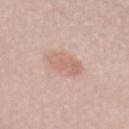workup — catalogued during a skin exam; not biopsied
TBP lesion metrics — a lesion area of about 8 mm², an eccentricity of roughly 0.65, and a shape-asymmetry score of about 0.25 (0 = symmetric); a color-variation rating of about 3/10 and a peripheral color-asymmetry measure near 1; a nevus-likeness score of about 10/100 and a lesion-detection confidence of about 100/100
diameter — about 3.5 mm
lighting — white-light illumination
image — 15 mm crop, total-body photography
site — the left forearm
patient — female, aged around 50